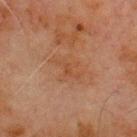{"biopsy_status": "not biopsied; imaged during a skin examination", "patient": {"sex": "male", "age_approx": 70}, "site": "chest", "lesion_size": {"long_diameter_mm_approx": 4.0}, "automated_metrics": {"area_mm2_approx": 8.5, "eccentricity": 0.75, "shape_asymmetry": 0.3, "cielab_L": 40, "cielab_a": 19, "cielab_b": 29, "vs_skin_contrast_norm": 5.0, "border_irregularity_0_10": 4.5, "color_variation_0_10": 3.0, "peripheral_color_asymmetry": 1.0}, "lighting": "cross-polarized", "image": {"source": "total-body photography crop", "field_of_view_mm": 15}}